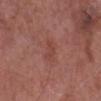Assessment: Part of a total-body skin-imaging series; this lesion was reviewed on a skin check and was not flagged for biopsy. Acquisition and patient details: Cropped from a total-body skin-imaging series; the visible field is about 15 mm. Imaged with white-light lighting. A male subject approximately 85 years of age or older. The lesion's longest dimension is about 3 mm. The total-body-photography lesion software estimated a lesion area of about 4.5 mm² and a shape eccentricity near 0.85. The software also gave a mean CIELAB color near L≈44 a*≈25 b*≈26, roughly 5 lightness units darker than nearby skin, and a normalized border contrast of about 4.5. The lesion is located on the left lower leg.